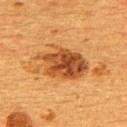Recorded during total-body skin imaging; not selected for excision or biopsy. The tile uses cross-polarized illumination. The lesion's longest dimension is about 6.5 mm. A female subject about 55 years old. From the upper back. A close-up tile cropped from a whole-body skin photograph, about 15 mm across. Automated tile analysis of the lesion measured an area of roughly 18 mm² and a shape-asymmetry score of about 0.25 (0 = symmetric). It also reported a mean CIELAB color near L≈43 a*≈24 b*≈39 and roughly 13 lightness units darker than nearby skin. The software also gave a border-irregularity rating of about 3/10 and peripheral color asymmetry of about 2.5. It also reported a nevus-likeness score of about 85/100 and lesion-presence confidence of about 100/100.